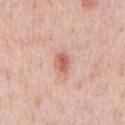Captured during whole-body skin photography for melanoma surveillance; the lesion was not biopsied. Captured under white-light illumination. A male patient, approximately 40 years of age. On the chest. A 15 mm close-up extracted from a 3D total-body photography capture. About 2.5 mm across.